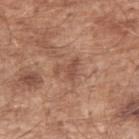Q: Was this lesion biopsied?
A: no biopsy performed (imaged during a skin exam)
Q: What is the imaging modality?
A: 15 mm crop, total-body photography
Q: What lighting was used for the tile?
A: white-light illumination
Q: Where on the body is the lesion?
A: the left upper arm
Q: Patient demographics?
A: male, aged 63–67
Q: How large is the lesion?
A: about 3 mm